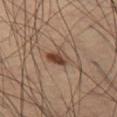| key | value |
|---|---|
| notes | catalogued during a skin exam; not biopsied |
| imaging modality | ~15 mm crop, total-body skin-cancer survey |
| lesion diameter | ~3 mm (longest diameter) |
| illumination | cross-polarized |
| patient | male, aged 58–62 |
| body site | the left thigh |